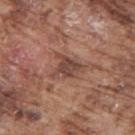Part of a total-body skin-imaging series; this lesion was reviewed on a skin check and was not flagged for biopsy. A male patient, aged 73–77. The total-body-photography lesion software estimated an area of roughly 7.5 mm², an eccentricity of roughly 0.65, and a symmetry-axis asymmetry near 0.5. And it measured an average lesion color of about L≈45 a*≈21 b*≈25 (CIELAB) and roughly 10 lightness units darker than nearby skin. It also reported a nevus-likeness score of about 5/100 and a detector confidence of about 90 out of 100 that the crop contains a lesion. The tile uses white-light illumination. The lesion is located on the upper back. The lesion's longest dimension is about 4 mm. A region of skin cropped from a whole-body photographic capture, roughly 15 mm wide.